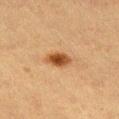From the leg.
A female subject about 55 years old.
A lesion tile, about 15 mm wide, cut from a 3D total-body photograph.